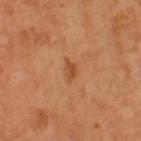The lesion was tiled from a total-body skin photograph and was not biopsied. About 3 mm across. A female subject, aged around 55. A 15 mm crop from a total-body photograph taken for skin-cancer surveillance. Automated tile analysis of the lesion measured a footprint of about 3 mm², a shape eccentricity near 0.85, and a symmetry-axis asymmetry near 0.35. The analysis additionally found a border-irregularity rating of about 3.5/10, a within-lesion color-variation index near 1.5/10, and a peripheral color-asymmetry measure near 0.5. The analysis additionally found a classifier nevus-likeness of about 60/100. The lesion is located on the left thigh.